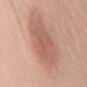Imaged during a routine full-body skin examination; the lesion was not biopsied and no histopathology is available. A roughly 15 mm field-of-view crop from a total-body skin photograph. About 6.5 mm across. The tile uses white-light illumination. The patient is a female in their mid-50s. An algorithmic analysis of the crop reported a lesion area of about 21 mm², a shape eccentricity near 0.75, and a shape-asymmetry score of about 0.2 (0 = symmetric). And it measured a lesion color around L≈58 a*≈23 b*≈28 in CIELAB, roughly 8 lightness units darker than nearby skin, and a normalized border contrast of about 5.5. On the arm.